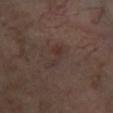No biopsy was performed on this lesion — it was imaged during a full skin examination and was not determined to be concerning.
About 4 mm across.
A roughly 15 mm field-of-view crop from a total-body skin photograph.
Automated image analysis of the tile measured a lesion area of about 4.5 mm², an eccentricity of roughly 0.9, and a shape-asymmetry score of about 0.5 (0 = symmetric). And it measured a lesion color around L≈32 a*≈15 b*≈19 in CIELAB and a lesion–skin lightness drop of about 5. The software also gave border irregularity of about 6.5 on a 0–10 scale and a peripheral color-asymmetry measure near 0.5.
Located on the left lower leg.
Captured under cross-polarized illumination.